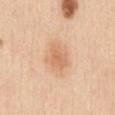notes: total-body-photography surveillance lesion; no biopsy | patient: female, aged approximately 30 | imaging modality: total-body-photography crop, ~15 mm field of view | illumination: white-light | diameter: ~4 mm (longest diameter) | site: the front of the torso.